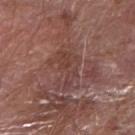{"biopsy_status": "not biopsied; imaged during a skin examination", "patient": {"sex": "male", "age_approx": 75}, "site": "arm", "image": {"source": "total-body photography crop", "field_of_view_mm": 15}}